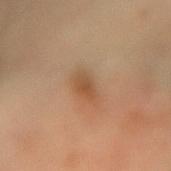Q: Is there a histopathology result?
A: total-body-photography surveillance lesion; no biopsy
Q: Where on the body is the lesion?
A: the left forearm
Q: What is the lesion's diameter?
A: ≈3 mm
Q: Illumination type?
A: cross-polarized
Q: What kind of image is this?
A: ~15 mm crop, total-body skin-cancer survey
Q: Patient demographics?
A: female, roughly 60 years of age